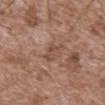biopsy_status: not biopsied; imaged during a skin examination
site: abdomen
lighting: white-light
automated_metrics:
  area_mm2_approx: 4.0
  shape_asymmetry: 0.35
  cielab_L: 49
  cielab_a: 20
  cielab_b: 28
  vs_skin_darker_L: 7.0
  vs_skin_contrast_norm: 5.5
image:
  source: total-body photography crop
  field_of_view_mm: 15
patient:
  sex: male
  age_approx: 75